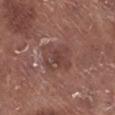The lesion was tiled from a total-body skin photograph and was not biopsied.
This image is a 15 mm lesion crop taken from a total-body photograph.
Imaged with white-light lighting.
Measured at roughly 4 mm in maximum diameter.
From the right lower leg.
The total-body-photography lesion software estimated a shape-asymmetry score of about 0.1 (0 = symmetric). It also reported a mean CIELAB color near L≈42 a*≈20 b*≈22, roughly 7 lightness units darker than nearby skin, and a lesion-to-skin contrast of about 6 (normalized; higher = more distinct). The analysis additionally found a border-irregularity index near 2/10 and a within-lesion color-variation index near 4/10. And it measured an automated nevus-likeness rating near 0 out of 100 and a detector confidence of about 100 out of 100 that the crop contains a lesion.
A male subject, in their mid- to late 70s.